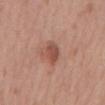No biopsy was performed on this lesion — it was imaged during a full skin examination and was not determined to be concerning.
The tile uses white-light illumination.
An algorithmic analysis of the crop reported a lesion area of about 5.5 mm², an outline eccentricity of about 0.8 (0 = round, 1 = elongated), and a symmetry-axis asymmetry near 0.2.
A 15 mm crop from a total-body photograph taken for skin-cancer surveillance.
Located on the mid back.
The patient is a female aged 43–47.